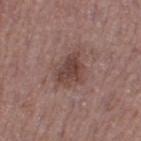Q: Was this lesion biopsied?
A: total-body-photography surveillance lesion; no biopsy
Q: How large is the lesion?
A: ~4 mm (longest diameter)
Q: Who is the patient?
A: female, aged 38–42
Q: How was the tile lit?
A: white-light
Q: What is the imaging modality?
A: ~15 mm tile from a whole-body skin photo
Q: Lesion location?
A: the left thigh
Q: Automated lesion metrics?
A: a footprint of about 8.5 mm², an eccentricity of roughly 0.6, and a symmetry-axis asymmetry near 0.35; a border-irregularity index near 3.5/10, a color-variation rating of about 3.5/10, and radial color variation of about 1; a classifier nevus-likeness of about 15/100 and a lesion-detection confidence of about 100/100